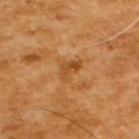Q: Was this lesion biopsied?
A: no biopsy performed (imaged during a skin exam)
Q: How was this image acquired?
A: total-body-photography crop, ~15 mm field of view
Q: Patient demographics?
A: male, aged around 65